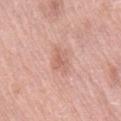workup = imaged on a skin check; not biopsied | imaging modality = ~15 mm tile from a whole-body skin photo | patient = female, approximately 70 years of age | body site = the leg.